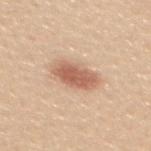- tile lighting — white-light illumination
- subject — female, approximately 25 years of age
- anatomic site — the mid back
- image — ~15 mm tile from a whole-body skin photo
- size — ~4.5 mm (longest diameter)
- TBP lesion metrics — a lesion area of about 11 mm² and an outline eccentricity of about 0.8 (0 = round, 1 = elongated); an average lesion color of about L≈61 a*≈22 b*≈32 (CIELAB), a lesion–skin lightness drop of about 13, and a normalized lesion–skin contrast near 8; a border-irregularity index near 2/10, internal color variation of about 4 on a 0–10 scale, and radial color variation of about 1.5; a nevus-likeness score of about 95/100 and lesion-presence confidence of about 100/100